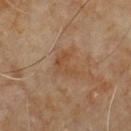Q: What kind of image is this?
A: 15 mm crop, total-body photography
Q: Lesion location?
A: the upper back
Q: Automated lesion metrics?
A: a footprint of about 6 mm², a shape eccentricity near 0.75, and two-axis asymmetry of about 0.5; a mean CIELAB color near L≈45 a*≈18 b*≈32, roughly 6 lightness units darker than nearby skin, and a normalized border contrast of about 5.5; a border-irregularity index near 5.5/10 and a within-lesion color-variation index near 3/10
Q: How large is the lesion?
A: ~3.5 mm (longest diameter)
Q: How was the tile lit?
A: cross-polarized
Q: Who is the patient?
A: male, in their 60s